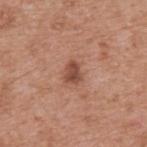Notes:
– notes · total-body-photography surveillance lesion; no biopsy
– site · the upper back
– image-analysis metrics · a lesion area of about 4 mm² and a shape eccentricity near 0.7; a border-irregularity index near 2.5/10, a color-variation rating of about 2.5/10, and peripheral color asymmetry of about 1
– lesion diameter · ≈2.5 mm
– imaging modality · 15 mm crop, total-body photography
– subject · male, about 55 years old